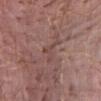{
  "biopsy_status": "not biopsied; imaged during a skin examination",
  "lesion_size": {
    "long_diameter_mm_approx": 3.5
  },
  "site": "abdomen",
  "automated_metrics": {
    "area_mm2_approx": 4.0,
    "eccentricity": 0.85,
    "shape_asymmetry": 0.8,
    "border_irregularity_0_10": 8.0,
    "peripheral_color_asymmetry": 0.0,
    "nevus_likeness_0_100": 0,
    "lesion_detection_confidence_0_100": 65
  },
  "patient": {
    "sex": "male",
    "age_approx": 40
  },
  "image": {
    "source": "total-body photography crop",
    "field_of_view_mm": 15
  },
  "lighting": "white-light"
}